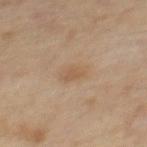notes = total-body-photography surveillance lesion; no biopsy
image = ~15 mm crop, total-body skin-cancer survey
automated metrics = a nevus-likeness score of about 0/100 and lesion-presence confidence of about 100/100
anatomic site = the mid back
subject = male, roughly 55 years of age
illumination = cross-polarized illumination